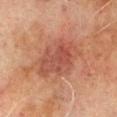Captured during whole-body skin photography for melanoma surveillance; the lesion was not biopsied.
Approximately 6 mm at its widest.
The total-body-photography lesion software estimated an area of roughly 20 mm² and a shape-asymmetry score of about 0.2 (0 = symmetric). The software also gave a lesion color around L≈42 a*≈23 b*≈26 in CIELAB, about 8 CIELAB-L* units darker than the surrounding skin, and a normalized lesion–skin contrast near 6.5. The software also gave a border-irregularity rating of about 2.5/10, internal color variation of about 4.5 on a 0–10 scale, and peripheral color asymmetry of about 1.5. The software also gave a lesion-detection confidence of about 100/100.
A lesion tile, about 15 mm wide, cut from a 3D total-body photograph.
A male subject, about 70 years old.
Captured under cross-polarized illumination.
The lesion is on the left lower leg.